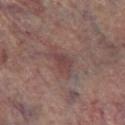workup = total-body-photography surveillance lesion; no biopsy
location = the right lower leg
image-analysis metrics = a lesion color around L≈43 a*≈20 b*≈19 in CIELAB, roughly 7 lightness units darker than nearby skin, and a normalized lesion–skin contrast near 6
patient = male, in their 70s
imaging modality = 15 mm crop, total-body photography